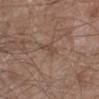biopsy status: total-body-photography surveillance lesion; no biopsy | subject: male, aged 58–62 | location: the leg | lesion size: ≈2.5 mm | imaging modality: ~15 mm crop, total-body skin-cancer survey | tile lighting: white-light | TBP lesion metrics: a border-irregularity index near 5/10 and radial color variation of about 0.